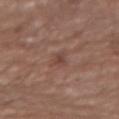notes: catalogued during a skin exam; not biopsied
image source: ~15 mm crop, total-body skin-cancer survey
patient: male, about 55 years old
lighting: white-light
size: ≈3 mm
site: the left forearm